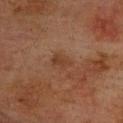Findings:
– notes · total-body-photography surveillance lesion; no biopsy
– patient · male, in their mid- to late 70s
– body site · the upper back
– illumination · cross-polarized
– imaging modality · ~15 mm crop, total-body skin-cancer survey